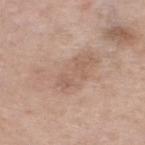The lesion was tiled from a total-body skin photograph and was not biopsied. Automated tile analysis of the lesion measured a lesion area of about 21 mm², a shape eccentricity near 0.85, and two-axis asymmetry of about 0.5. It also reported a classifier nevus-likeness of about 0/100 and a detector confidence of about 95 out of 100 that the crop contains a lesion. Measured at roughly 8.5 mm in maximum diameter. The lesion is located on the left lower leg. A female subject, in their mid- to late 50s. Imaged with white-light lighting. A lesion tile, about 15 mm wide, cut from a 3D total-body photograph.